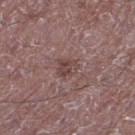Imaged with white-light lighting. The subject is a male in their 50s. This image is a 15 mm lesion crop taken from a total-body photograph. The lesion is located on the right lower leg. Automated image analysis of the tile measured a lesion area of about 4.5 mm² and an eccentricity of roughly 0.55. The analysis additionally found an average lesion color of about L≈43 a*≈19 b*≈19 (CIELAB), roughly 8 lightness units darker than nearby skin, and a normalized border contrast of about 6.5. The analysis additionally found a nevus-likeness score of about 0/100. About 2.5 mm across.